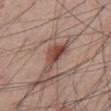{"biopsy_status": "not biopsied; imaged during a skin examination", "lesion_size": {"long_diameter_mm_approx": 4.5}, "image": {"source": "total-body photography crop", "field_of_view_mm": 15}, "site": "abdomen", "automated_metrics": {"area_mm2_approx": 7.0, "eccentricity": 0.85, "shape_asymmetry": 0.5, "nevus_likeness_0_100": 70, "lesion_detection_confidence_0_100": 100}, "lighting": "white-light", "patient": {"sex": "male", "age_approx": 45}}